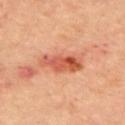notes = no biopsy performed (imaged during a skin exam)
imaging modality = ~15 mm crop, total-body skin-cancer survey
subject = male, roughly 60 years of age
site = the upper back
illumination = cross-polarized illumination